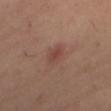Acquisition and patient details: The subject is a female about 40 years old. The lesion's longest dimension is about 3 mm. On the right thigh. A roughly 15 mm field-of-view crop from a total-body skin photograph. Captured under cross-polarized illumination.